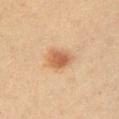follow-up — catalogued during a skin exam; not biopsied
automated metrics — a lesion–skin lightness drop of about 11 and a normalized border contrast of about 8; a border-irregularity index near 2.5/10, a within-lesion color-variation index near 3/10, and peripheral color asymmetry of about 1
patient — male, aged approximately 40
anatomic site — the arm
tile lighting — cross-polarized
image — ~15 mm crop, total-body skin-cancer survey
diameter — about 3.5 mm